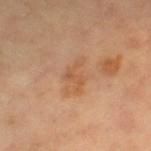Part of a total-body skin-imaging series; this lesion was reviewed on a skin check and was not flagged for biopsy.